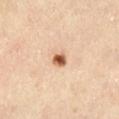The lesion's longest dimension is about 2 mm. The subject is a male aged 63 to 67. From the leg. The tile uses cross-polarized illumination. An algorithmic analysis of the crop reported a footprint of about 3 mm², an outline eccentricity of about 0.5 (0 = round, 1 = elongated), and a shape-asymmetry score of about 0.25 (0 = symmetric). A close-up tile cropped from a whole-body skin photograph, about 15 mm across.